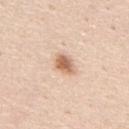Captured during whole-body skin photography for melanoma surveillance; the lesion was not biopsied. The lesion-visualizer software estimated a border-irregularity rating of about 2/10 and a within-lesion color-variation index near 3/10. Captured under white-light illumination. Located on the mid back. A 15 mm close-up tile from a total-body photography series done for melanoma screening. A male patient roughly 45 years of age. The lesion's longest dimension is about 2.5 mm.